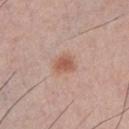Imaged during a routine full-body skin examination; the lesion was not biopsied and no histopathology is available.